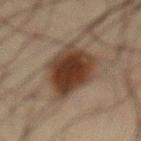A male subject aged 58–62. Longest diameter approximately 6.5 mm. From the front of the torso. A roughly 15 mm field-of-view crop from a total-body skin photograph. Automated image analysis of the tile measured an area of roughly 21 mm², an outline eccentricity of about 0.75 (0 = round, 1 = elongated), and a shape-asymmetry score of about 0.2 (0 = symmetric). It also reported roughly 12 lightness units darker than nearby skin and a lesion-to-skin contrast of about 12.5 (normalized; higher = more distinct). It also reported a nevus-likeness score of about 100/100 and lesion-presence confidence of about 100/100.